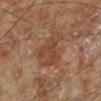Part of a total-body skin-imaging series; this lesion was reviewed on a skin check and was not flagged for biopsy. Captured under cross-polarized illumination. Located on the left lower leg. Cropped from a total-body skin-imaging series; the visible field is about 15 mm. A male subject about 70 years old. The total-body-photography lesion software estimated a lesion color around L≈43 a*≈20 b*≈30 in CIELAB, about 7 CIELAB-L* units darker than the surrounding skin, and a normalized lesion–skin contrast near 6. The software also gave border irregularity of about 4 on a 0–10 scale. The analysis additionally found a classifier nevus-likeness of about 0/100 and a detector confidence of about 100 out of 100 that the crop contains a lesion.